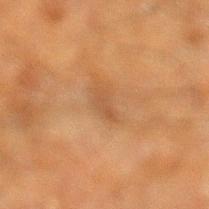{"biopsy_status": "not biopsied; imaged during a skin examination", "patient": {"sex": "male", "age_approx": 60}, "automated_metrics": {"color_variation_0_10": 0.5, "nevus_likeness_0_100": 0, "lesion_detection_confidence_0_100": 95}, "image": {"source": "total-body photography crop", "field_of_view_mm": 15}, "lesion_size": {"long_diameter_mm_approx": 3.0}, "site": "leg", "lighting": "cross-polarized"}